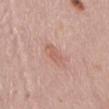Clinical impression: Part of a total-body skin-imaging series; this lesion was reviewed on a skin check and was not flagged for biopsy. Acquisition and patient details: The lesion's longest dimension is about 3 mm. From the abdomen. Imaged with white-light lighting. A female subject, in their mid- to late 60s. A 15 mm close-up extracted from a 3D total-body photography capture. The total-body-photography lesion software estimated an eccentricity of roughly 0.85 and a shape-asymmetry score of about 0.2 (0 = symmetric). It also reported a mean CIELAB color near L≈60 a*≈22 b*≈27. The software also gave an automated nevus-likeness rating near 5 out of 100.